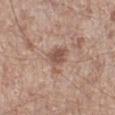notes: no biopsy performed (imaged during a skin exam)
size: about 3.5 mm
anatomic site: the left lower leg
patient: male, aged approximately 55
lighting: white-light
image source: ~15 mm crop, total-body skin-cancer survey